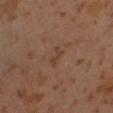* workup — imaged on a skin check; not biopsied
* location — the left lower leg
* lighting — cross-polarized illumination
* subject — male, in their 30s
* diameter — about 2.5 mm
* imaging modality — total-body-photography crop, ~15 mm field of view